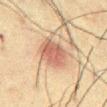Recorded during total-body skin imaging; not selected for excision or biopsy. This image is a 15 mm lesion crop taken from a total-body photograph. The subject is a male aged 58–62. The tile uses cross-polarized illumination. From the chest.